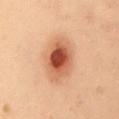Case summary:
* follow-up — imaged on a skin check; not biopsied
* body site — the chest
* illumination — cross-polarized
* patient — female, aged approximately 40
* lesion size — ~5 mm (longest diameter)
* image source — total-body-photography crop, ~15 mm field of view